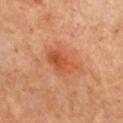Assessment:
The lesion was tiled from a total-body skin photograph and was not biopsied.
Image and clinical context:
Automated tile analysis of the lesion measured a footprint of about 9 mm² and a shape eccentricity near 0.8. And it measured an average lesion color of about L≈52 a*≈29 b*≈38 (CIELAB), about 9 CIELAB-L* units darker than the surrounding skin, and a lesion-to-skin contrast of about 6.5 (normalized; higher = more distinct). The software also gave a color-variation rating of about 5/10. The software also gave an automated nevus-likeness rating near 85 out of 100. The lesion is located on the chest. The tile uses cross-polarized illumination. A lesion tile, about 15 mm wide, cut from a 3D total-body photograph. The subject is a female in their mid-60s.